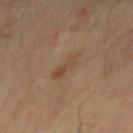The lesion was tiled from a total-body skin photograph and was not biopsied. The lesion is located on the mid back. The tile uses cross-polarized illumination. A 15 mm close-up extracted from a 3D total-body photography capture. Longest diameter approximately 4 mm. Automated tile analysis of the lesion measured an area of roughly 5 mm² and an outline eccentricity of about 0.95 (0 = round, 1 = elongated). The software also gave an average lesion color of about L≈36 a*≈13 b*≈25 (CIELAB), about 5 CIELAB-L* units darker than the surrounding skin, and a lesion-to-skin contrast of about 5.5 (normalized; higher = more distinct). A male patient approximately 70 years of age.